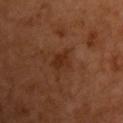The lesion was tiled from a total-body skin photograph and was not biopsied.